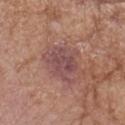This lesion was catalogued during total-body skin photography and was not selected for biopsy. Located on the left upper arm. The tile uses white-light illumination. Automated image analysis of the tile measured an area of roughly 13 mm², a shape eccentricity near 0.65, and two-axis asymmetry of about 0.35. And it measured a lesion color around L≈48 a*≈22 b*≈20 in CIELAB and a normalized lesion–skin contrast near 8. It also reported border irregularity of about 4.5 on a 0–10 scale, a within-lesion color-variation index near 3.5/10, and a peripheral color-asymmetry measure near 1. The software also gave a detector confidence of about 100 out of 100 that the crop contains a lesion. About 5 mm across. A male patient roughly 85 years of age or older. Cropped from a total-body skin-imaging series; the visible field is about 15 mm.